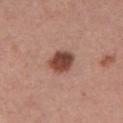Impression:
Captured during whole-body skin photography for melanoma surveillance; the lesion was not biopsied.
Background:
A male patient, in their 40s. The lesion-visualizer software estimated a lesion area of about 7.5 mm², an eccentricity of roughly 0.4, and a shape-asymmetry score of about 0.1 (0 = symmetric). And it measured a classifier nevus-likeness of about 100/100 and a detector confidence of about 100 out of 100 that the crop contains a lesion. A region of skin cropped from a whole-body photographic capture, roughly 15 mm wide. This is a white-light tile. About 3 mm across. The lesion is located on the chest.